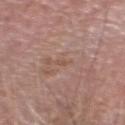About 1.5 mm across.
The lesion is on the head or neck.
The subject is a male approximately 65 years of age.
Cropped from a total-body skin-imaging series; the visible field is about 15 mm.